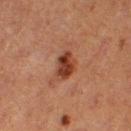Clinical impression:
No biopsy was performed on this lesion — it was imaged during a full skin examination and was not determined to be concerning.
Clinical summary:
The lesion's longest dimension is about 3 mm. A close-up tile cropped from a whole-body skin photograph, about 15 mm across. Located on the right thigh. Imaged with cross-polarized lighting. A female subject aged around 40.